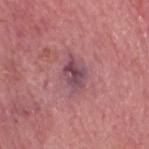notes: no biopsy performed (imaged during a skin exam)
lesion size: ~4.5 mm (longest diameter)
image source: ~15 mm crop, total-body skin-cancer survey
site: the head or neck
TBP lesion metrics: an area of roughly 8.5 mm², an outline eccentricity of about 0.75 (0 = round, 1 = elongated), and two-axis asymmetry of about 0.2; a lesion–skin lightness drop of about 10 and a normalized lesion–skin contrast near 8.5; a border-irregularity rating of about 2.5/10 and radial color variation of about 2.5; an automated nevus-likeness rating near 0 out of 100 and a lesion-detection confidence of about 100/100
subject: male, in their mid-60s
illumination: white-light illumination